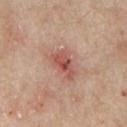Background: The lesion is on the front of the torso. A male patient about 65 years old. Measured at roughly 4 mm in maximum diameter. A 15 mm close-up tile from a total-body photography series done for melanoma screening. Captured under white-light illumination.